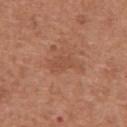| field | value |
|---|---|
| follow-up | imaged on a skin check; not biopsied |
| subject | male, approximately 65 years of age |
| body site | the abdomen |
| imaging modality | total-body-photography crop, ~15 mm field of view |